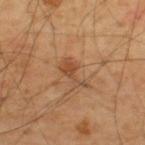Notes:
- biopsy status · no biopsy performed (imaged during a skin exam)
- image source · total-body-photography crop, ~15 mm field of view
- lighting · cross-polarized illumination
- lesion diameter · about 4 mm
- site · the upper back
- subject · male, approximately 55 years of age